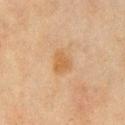Clinical impression: The lesion was tiled from a total-body skin photograph and was not biopsied. Image and clinical context: The lesion is located on the chest. The subject is a male aged 68 to 72. A roughly 15 mm field-of-view crop from a total-body skin photograph.